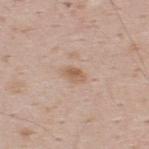Q: Was a biopsy performed?
A: imaged on a skin check; not biopsied
Q: Who is the patient?
A: male, approximately 35 years of age
Q: Automated lesion metrics?
A: about 9 CIELAB-L* units darker than the surrounding skin and a lesion-to-skin contrast of about 7 (normalized; higher = more distinct); a border-irregularity rating of about 2/10; a nevus-likeness score of about 30/100 and a lesion-detection confidence of about 100/100
Q: How was this image acquired?
A: 15 mm crop, total-body photography
Q: Lesion size?
A: about 2.5 mm
Q: Where on the body is the lesion?
A: the upper back